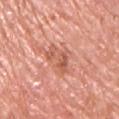<tbp_lesion>
  <biopsy_status>not biopsied; imaged during a skin examination</biopsy_status>
  <image>
    <source>total-body photography crop</source>
    <field_of_view_mm>15</field_of_view_mm>
  </image>
  <site>chest</site>
  <patient>
    <sex>male</sex>
    <age_approx>70</age_approx>
  </patient>
  <lighting>white-light</lighting>
</tbp_lesion>